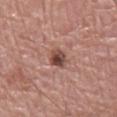Captured during whole-body skin photography for melanoma surveillance; the lesion was not biopsied. Captured under white-light illumination. A lesion tile, about 15 mm wide, cut from a 3D total-body photograph. Automated tile analysis of the lesion measured an area of roughly 4.5 mm², a shape eccentricity near 0.65, and two-axis asymmetry of about 0.2. The software also gave a color-variation rating of about 6/10 and radial color variation of about 2. Measured at roughly 3 mm in maximum diameter. A male patient, aged 68 to 72. The lesion is on the leg.